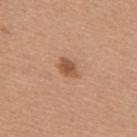Q: Is there a histopathology result?
A: imaged on a skin check; not biopsied
Q: What is the anatomic site?
A: the upper back
Q: What are the patient's age and sex?
A: female, aged around 45
Q: What kind of image is this?
A: ~15 mm tile from a whole-body skin photo
Q: Illumination type?
A: white-light illumination